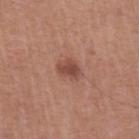{
  "biopsy_status": "not biopsied; imaged during a skin examination",
  "lesion_size": {
    "long_diameter_mm_approx": 3.0
  },
  "patient": {
    "sex": "male",
    "age_approx": 65
  },
  "lighting": "white-light",
  "site": "chest",
  "image": {
    "source": "total-body photography crop",
    "field_of_view_mm": 15
  }
}